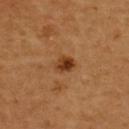Impression:
Imaged during a routine full-body skin examination; the lesion was not biopsied and no histopathology is available.
Context:
Located on the upper back. Measured at roughly 2.5 mm in maximum diameter. Automated tile analysis of the lesion measured a footprint of about 5 mm², a shape eccentricity near 0.6, and a shape-asymmetry score of about 0.25 (0 = symmetric). It also reported roughly 12 lightness units darker than nearby skin and a normalized border contrast of about 9. It also reported an automated nevus-likeness rating near 95 out of 100 and lesion-presence confidence of about 100/100. A female patient aged approximately 60. Captured under cross-polarized illumination. A 15 mm close-up extracted from a 3D total-body photography capture.